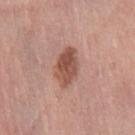{"site": "right thigh", "patient": {"sex": "female", "age_approx": 65}, "image": {"source": "total-body photography crop", "field_of_view_mm": 15}, "lighting": "white-light", "lesion_size": {"long_diameter_mm_approx": 4.5}}